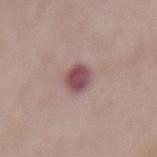{"biopsy_status": "not biopsied; imaged during a skin examination", "site": "mid back", "lighting": "white-light", "automated_metrics": {"cielab_L": 49, "cielab_a": 23, "cielab_b": 16, "vs_skin_darker_L": 14.0, "vs_skin_contrast_norm": 10.5, "border_irregularity_0_10": 1.5, "color_variation_0_10": 4.5, "peripheral_color_asymmetry": 1.5, "nevus_likeness_0_100": 10}, "image": {"source": "total-body photography crop", "field_of_view_mm": 15}, "lesion_size": {"long_diameter_mm_approx": 3.5}, "patient": {"sex": "female", "age_approx": 70}}